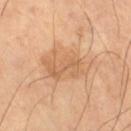Impression:
Imaged during a routine full-body skin examination; the lesion was not biopsied and no histopathology is available.
Clinical summary:
Cropped from a total-body skin-imaging series; the visible field is about 15 mm. The lesion is on the leg. The patient is a male aged 63 to 67.